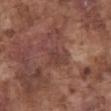Recorded during total-body skin imaging; not selected for excision or biopsy. Automated tile analysis of the lesion measured radial color variation of about 0.5. And it measured an automated nevus-likeness rating near 0 out of 100 and lesion-presence confidence of about 75/100. Imaged with white-light lighting. This image is a 15 mm lesion crop taken from a total-body photograph. Located on the abdomen. About 4.5 mm across. A male patient, aged 73 to 77.